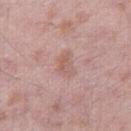No biopsy was performed on this lesion — it was imaged during a full skin examination and was not determined to be concerning. The patient is a male aged approximately 45. The lesion-visualizer software estimated an average lesion color of about L≈59 a*≈20 b*≈24 (CIELAB), a lesion–skin lightness drop of about 7, and a normalized border contrast of about 5. The tile uses white-light illumination. The lesion is on the leg. Measured at roughly 3.5 mm in maximum diameter. A 15 mm close-up extracted from a 3D total-body photography capture.